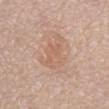Assessment: This lesion was catalogued during total-body skin photography and was not selected for biopsy. Background: This is a white-light tile. A 15 mm close-up extracted from a 3D total-body photography capture. The recorded lesion diameter is about 5.5 mm. Located on the abdomen. The subject is a female in their mid- to late 60s.